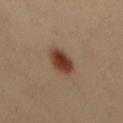follow-up: catalogued during a skin exam; not biopsied
acquisition: ~15 mm tile from a whole-body skin photo
body site: the mid back
patient: male, aged 38–42
diameter: ~3.5 mm (longest diameter)